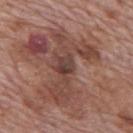- workup: total-body-photography surveillance lesion; no biopsy
- TBP lesion metrics: a footprint of about 24 mm² and a shape eccentricity near 0.8; a lesion color around L≈43 a*≈21 b*≈24 in CIELAB; a border-irregularity rating of about 9.5/10, a within-lesion color-variation index near 5.5/10, and a peripheral color-asymmetry measure near 1.5
- tile lighting: white-light illumination
- acquisition: total-body-photography crop, ~15 mm field of view
- lesion size: ≈8 mm
- anatomic site: the mid back
- patient: male, roughly 70 years of age